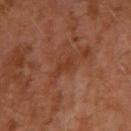Impression: This lesion was catalogued during total-body skin photography and was not selected for biopsy. Context: From the left arm. This is a cross-polarized tile. Approximately 3.5 mm at its widest. A close-up tile cropped from a whole-body skin photograph, about 15 mm across. A male patient aged 28 to 32.